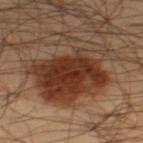This lesion was catalogued during total-body skin photography and was not selected for biopsy.
A male subject, aged 43 to 47.
The total-body-photography lesion software estimated a lesion area of about 43 mm², an eccentricity of roughly 0.5, and a shape-asymmetry score of about 0.3 (0 = symmetric). And it measured an average lesion color of about L≈35 a*≈20 b*≈28 (CIELAB), roughly 11 lightness units darker than nearby skin, and a normalized border contrast of about 10.
A 15 mm close-up tile from a total-body photography series done for melanoma screening.
The recorded lesion diameter is about 8.5 mm.
Captured under cross-polarized illumination.
On the right lower leg.